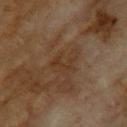Q: Was a biopsy performed?
A: total-body-photography surveillance lesion; no biopsy
Q: What did automated image analysis measure?
A: a color-variation rating of about 0/10 and radial color variation of about 0; a detector confidence of about 100 out of 100 that the crop contains a lesion
Q: Who is the patient?
A: female, roughly 60 years of age
Q: Lesion location?
A: the upper back
Q: What is the imaging modality?
A: ~15 mm tile from a whole-body skin photo
Q: Illumination type?
A: cross-polarized
Q: What is the lesion's diameter?
A: ~3 mm (longest diameter)